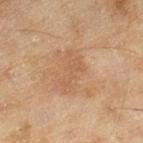follow-up: total-body-photography surveillance lesion; no biopsy | image: 15 mm crop, total-body photography | lesion size: about 4.5 mm | patient: female, in their 60s | body site: the right lower leg | TBP lesion metrics: a footprint of about 7 mm², a shape eccentricity near 0.9, and a symmetry-axis asymmetry near 0.4; roughly 6 lightness units darker than nearby skin and a normalized lesion–skin contrast near 4.5; internal color variation of about 1.5 on a 0–10 scale and peripheral color asymmetry of about 0.5; a nevus-likeness score of about 0/100 and lesion-presence confidence of about 100/100.